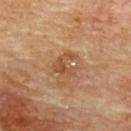Clinical impression: Part of a total-body skin-imaging series; this lesion was reviewed on a skin check and was not flagged for biopsy. Acquisition and patient details: The subject is a male aged 83 or older. Located on the upper back. Imaged with cross-polarized lighting. The recorded lesion diameter is about 3 mm. A 15 mm crop from a total-body photograph taken for skin-cancer surveillance.